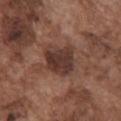Notes:
• workup: imaged on a skin check; not biopsied
• image source: ~15 mm tile from a whole-body skin photo
• body site: the front of the torso
• subject: male, aged 73–77